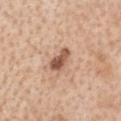Impression:
The lesion was tiled from a total-body skin photograph and was not biopsied.
Acquisition and patient details:
On the head or neck. Captured under white-light illumination. A female subject aged 68–72. A 15 mm close-up extracted from a 3D total-body photography capture. Automated image analysis of the tile measured a normalized border contrast of about 9.5. The analysis additionally found a border-irregularity index near 3/10, a within-lesion color-variation index near 5/10, and radial color variation of about 1.5. The software also gave an automated nevus-likeness rating near 80 out of 100 and a detector confidence of about 100 out of 100 that the crop contains a lesion. About 3.5 mm across.